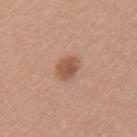Notes:
• notes: total-body-photography surveillance lesion; no biopsy
• site: the left upper arm
• illumination: white-light
• image source: ~15 mm tile from a whole-body skin photo
• TBP lesion metrics: a mean CIELAB color near L≈54 a*≈22 b*≈30, roughly 11 lightness units darker than nearby skin, and a normalized border contrast of about 7.5; internal color variation of about 2.5 on a 0–10 scale and a peripheral color-asymmetry measure near 0.5; a classifier nevus-likeness of about 95/100
• subject: female, roughly 35 years of age